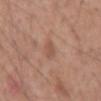– follow-up: total-body-photography surveillance lesion; no biopsy
– tile lighting: white-light illumination
– body site: the back
– size: about 2.5 mm
– patient: male, aged around 55
– image: ~15 mm tile from a whole-body skin photo